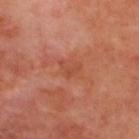Clinical impression: Part of a total-body skin-imaging series; this lesion was reviewed on a skin check and was not flagged for biopsy. Acquisition and patient details: A male patient aged 48–52. Located on the upper back. A close-up tile cropped from a whole-body skin photograph, about 15 mm across.